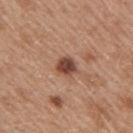  biopsy_status: not biopsied; imaged during a skin examination
  lighting: white-light
  patient:
    sex: female
    age_approx: 40
  image:
    source: total-body photography crop
    field_of_view_mm: 15
  site: arm